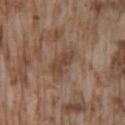Captured during whole-body skin photography for melanoma surveillance; the lesion was not biopsied. Cropped from a whole-body photographic skin survey; the tile spans about 15 mm. A male patient, in their mid- to late 70s. On the lower back.